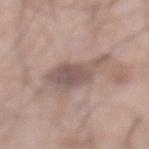patient=male, aged 68 to 72 | automated metrics=a lesion color around L≈54 a*≈15 b*≈20 in CIELAB and about 10 CIELAB-L* units darker than the surrounding skin; border irregularity of about 4.5 on a 0–10 scale and a peripheral color-asymmetry measure near 1 | image source=~15 mm tile from a whole-body skin photo | illumination=white-light | lesion size=about 5.5 mm | location=the abdomen.